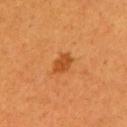No biopsy was performed on this lesion — it was imaged during a full skin examination and was not determined to be concerning. A 15 mm close-up extracted from a 3D total-body photography capture. From the left upper arm. Approximately 3 mm at its widest. An algorithmic analysis of the crop reported a border-irregularity index near 2/10 and internal color variation of about 1.5 on a 0–10 scale. And it measured a nevus-likeness score of about 95/100 and lesion-presence confidence of about 100/100. Captured under cross-polarized illumination. The subject is a female about 30 years old.